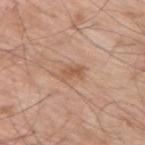Impression: The lesion was photographed on a routine skin check and not biopsied; there is no pathology result. Image and clinical context: Cropped from a total-body skin-imaging series; the visible field is about 15 mm. Measured at roughly 3 mm in maximum diameter. An algorithmic analysis of the crop reported a mean CIELAB color near L≈57 a*≈21 b*≈32, about 8 CIELAB-L* units darker than the surrounding skin, and a normalized lesion–skin contrast near 6. A male patient, aged around 70. Located on the back. This is a white-light tile.